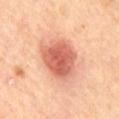Clinical impression:
Captured during whole-body skin photography for melanoma surveillance; the lesion was not biopsied.
Image and clinical context:
The lesion is located on the front of the torso. A male subject about 65 years old. A 15 mm close-up tile from a total-body photography series done for melanoma screening.